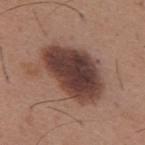Part of a total-body skin-imaging series; this lesion was reviewed on a skin check and was not flagged for biopsy. A male patient approximately 65 years of age. The lesion is on the back. Cropped from a whole-body photographic skin survey; the tile spans about 15 mm. Automated image analysis of the tile measured an automated nevus-likeness rating near 50 out of 100 and a detector confidence of about 100 out of 100 that the crop contains a lesion. Approximately 8.5 mm at its widest. This is a white-light tile.